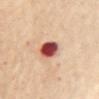This lesion was catalogued during total-body skin photography and was not selected for biopsy. The lesion is located on the chest. The subject is a female aged approximately 60. The tile uses cross-polarized illumination. The total-body-photography lesion software estimated a footprint of about 7 mm² and a shape eccentricity near 0.6. The analysis additionally found an average lesion color of about L≈50 a*≈32 b*≈28 (CIELAB) and a lesion–skin lightness drop of about 24. And it measured border irregularity of about 1 on a 0–10 scale, a color-variation rating of about 6.5/10, and radial color variation of about 2. Approximately 3.5 mm at its widest. Cropped from a whole-body photographic skin survey; the tile spans about 15 mm.